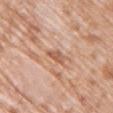| field | value |
|---|---|
| workup | total-body-photography surveillance lesion; no biopsy |
| image source | ~15 mm tile from a whole-body skin photo |
| subject | female, aged around 70 |
| illumination | white-light |
| body site | the front of the torso |
| lesion diameter | about 3.5 mm |